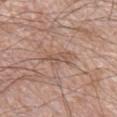Part of a total-body skin-imaging series; this lesion was reviewed on a skin check and was not flagged for biopsy. The tile uses white-light illumination. A region of skin cropped from a whole-body photographic capture, roughly 15 mm wide. The lesion is located on the right lower leg. A male subject, aged 58–62. The lesion's longest dimension is about 3.5 mm.